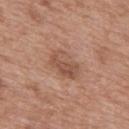workup = catalogued during a skin exam; not biopsied
TBP lesion metrics = a classifier nevus-likeness of about 10/100 and lesion-presence confidence of about 100/100
diameter = ≈4 mm
body site = the mid back
patient = male, aged approximately 50
lighting = white-light illumination
image source = ~15 mm tile from a whole-body skin photo